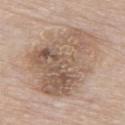Clinical impression:
The lesion was photographed on a routine skin check and not biopsied; there is no pathology result.
Context:
The patient is a male in their mid-80s or older. The lesion is located on the upper back. A close-up tile cropped from a whole-body skin photograph, about 15 mm across.